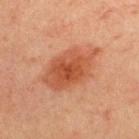Clinical impression: No biopsy was performed on this lesion — it was imaged during a full skin examination and was not determined to be concerning. Background: The lesion is located on the back. An algorithmic analysis of the crop reported border irregularity of about 3 on a 0–10 scale, a within-lesion color-variation index near 4/10, and peripheral color asymmetry of about 1. A male patient aged approximately 60. A roughly 15 mm field-of-view crop from a total-body skin photograph.